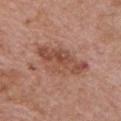| key | value |
|---|---|
| follow-up | no biopsy performed (imaged during a skin exam) |
| patient | male, about 70 years old |
| body site | the chest |
| imaging modality | total-body-photography crop, ~15 mm field of view |